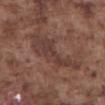The subject is a male about 75 years old. Automated image analysis of the tile measured an outline eccentricity of about 0.95 (0 = round, 1 = elongated) and a shape-asymmetry score of about 0.5 (0 = symmetric). And it measured a nevus-likeness score of about 0/100 and lesion-presence confidence of about 80/100. A region of skin cropped from a whole-body photographic capture, roughly 15 mm wide. From the abdomen.